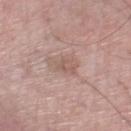Case summary:
• follow-up · imaged on a skin check; not biopsied
• illumination · white-light
• body site · the right lower leg
• lesion diameter · ~2.5 mm (longest diameter)
• subject · male, about 70 years old
• acquisition · ~15 mm crop, total-body skin-cancer survey